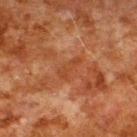Imaged during a routine full-body skin examination; the lesion was not biopsied and no histopathology is available. The lesion is located on the upper back. The lesion's longest dimension is about 3.5 mm. A male patient in their 80s. Automated image analysis of the tile measured a mean CIELAB color near L≈35 a*≈23 b*≈31 and roughly 5 lightness units darker than nearby skin. It also reported a border-irregularity rating of about 4/10 and a peripheral color-asymmetry measure near 0.5. The software also gave a classifier nevus-likeness of about 0/100 and a lesion-detection confidence of about 100/100. This image is a 15 mm lesion crop taken from a total-body photograph.